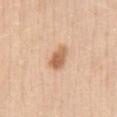biopsy status — imaged on a skin check; not biopsied | patient — female, aged around 30 | image — 15 mm crop, total-body photography | anatomic site — the abdomen | illumination — white-light illumination | automated lesion analysis — a shape eccentricity near 0.8 and a shape-asymmetry score of about 0.2 (0 = symmetric); border irregularity of about 2 on a 0–10 scale, a color-variation rating of about 4/10, and radial color variation of about 1 | size — ~3.5 mm (longest diameter).